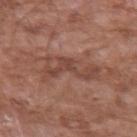This lesion was catalogued during total-body skin photography and was not selected for biopsy. Captured under white-light illumination. Approximately 6.5 mm at its widest. A region of skin cropped from a whole-body photographic capture, roughly 15 mm wide. The lesion is located on the left upper arm. A male patient, aged 58 to 62.